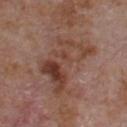  biopsy_status: not biopsied; imaged during a skin examination
  image:
    source: total-body photography crop
    field_of_view_mm: 15
  patient:
    sex: male
    age_approx: 65
  site: chest
  lesion_size:
    long_diameter_mm_approx: 7.5
  automated_metrics:
    eccentricity: 0.8
    shape_asymmetry: 0.45
    lesion_detection_confidence_0_100: 100